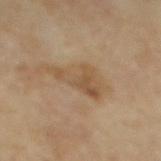{
  "biopsy_status": "not biopsied; imaged during a skin examination",
  "site": "mid back",
  "lesion_size": {
    "long_diameter_mm_approx": 5.5
  },
  "lighting": "cross-polarized",
  "image": {
    "source": "total-body photography crop",
    "field_of_view_mm": 15
  },
  "patient": {
    "sex": "male",
    "age_approx": "85+"
  },
  "automated_metrics": {
    "eccentricity": 0.85,
    "shape_asymmetry": 0.6
  }
}